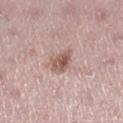follow-up=catalogued during a skin exam; not biopsied
image source=total-body-photography crop, ~15 mm field of view
patient=female, aged approximately 45
anatomic site=the right lower leg
diameter=about 3.5 mm